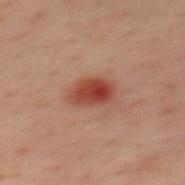Q: What is the imaging modality?
A: ~15 mm tile from a whole-body skin photo
Q: Lesion location?
A: the upper back
Q: Patient demographics?
A: male, aged around 40
Q: How large is the lesion?
A: ≈3.5 mm
Q: What lighting was used for the tile?
A: cross-polarized